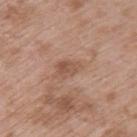biopsy status: total-body-photography surveillance lesion; no biopsy | subject: male, in their 50s | anatomic site: the upper back | acquisition: ~15 mm tile from a whole-body skin photo | lighting: white-light.